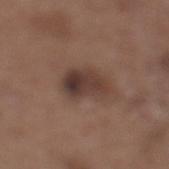Case summary:
* biopsy status · imaged on a skin check; not biopsied
* body site · the leg
* diameter · ~5 mm (longest diameter)
* image source · ~15 mm crop, total-body skin-cancer survey
* lighting · white-light illumination
* subject · male, approximately 65 years of age
* automated metrics · a lesion color around L≈37 a*≈17 b*≈22 in CIELAB, about 11 CIELAB-L* units darker than the surrounding skin, and a lesion-to-skin contrast of about 9.5 (normalized; higher = more distinct); a lesion-detection confidence of about 100/100